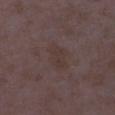{"biopsy_status": "not biopsied; imaged during a skin examination", "lighting": "white-light", "patient": {"sex": "female", "age_approx": 35}, "site": "right lower leg", "image": {"source": "total-body photography crop", "field_of_view_mm": 15}, "lesion_size": {"long_diameter_mm_approx": 3.5}}